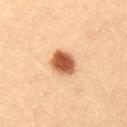Captured during whole-body skin photography for melanoma surveillance; the lesion was not biopsied.
The recorded lesion diameter is about 3.5 mm.
The lesion is on the lower back.
A female patient aged approximately 30.
The total-body-photography lesion software estimated a border-irregularity rating of about 1.5/10 and peripheral color asymmetry of about 1.5.
A region of skin cropped from a whole-body photographic capture, roughly 15 mm wide.